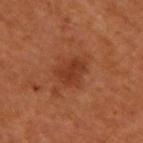Assessment: This lesion was catalogued during total-body skin photography and was not selected for biopsy. Context: Approximately 3.5 mm at its widest. This image is a 15 mm lesion crop taken from a total-body photograph. Located on the upper back. A male subject, in their 50s. The lesion-visualizer software estimated a footprint of about 8 mm², a shape eccentricity near 0.6, and two-axis asymmetry of about 0.25. The software also gave border irregularity of about 2.5 on a 0–10 scale, a within-lesion color-variation index near 2.5/10, and peripheral color asymmetry of about 1. It also reported lesion-presence confidence of about 100/100. The tile uses cross-polarized illumination.